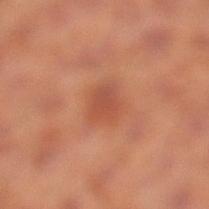{
  "biopsy_status": "not biopsied; imaged during a skin examination",
  "site": "left lower leg",
  "lesion_size": {
    "long_diameter_mm_approx": 3.0
  },
  "patient": {
    "sex": "male",
    "age_approx": 65
  },
  "image": {
    "source": "total-body photography crop",
    "field_of_view_mm": 15
  },
  "lighting": "cross-polarized"
}